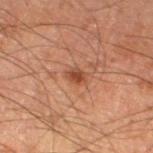notes: total-body-photography surveillance lesion; no biopsy | lesion size: about 2.5 mm | tile lighting: cross-polarized illumination | automated metrics: a mean CIELAB color near L≈43 a*≈24 b*≈31, a lesion–skin lightness drop of about 10, and a lesion-to-skin contrast of about 7.5 (normalized; higher = more distinct); border irregularity of about 1.5 on a 0–10 scale, a within-lesion color-variation index near 2.5/10, and peripheral color asymmetry of about 1 | subject: male, approximately 60 years of age | body site: the left thigh | image source: total-body-photography crop, ~15 mm field of view.